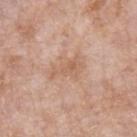<case>
  <image>
    <source>total-body photography crop</source>
    <field_of_view_mm>15</field_of_view_mm>
  </image>
  <site>left forearm</site>
  <automated_metrics>
    <lesion_detection_confidence_0_100>100</lesion_detection_confidence_0_100>
  </automated_metrics>
  <patient>
    <sex>female</sex>
    <age_approx>70</age_approx>
  </patient>
  <lesion_size>
    <long_diameter_mm_approx>4.0</long_diameter_mm_approx>
  </lesion_size>
</case>